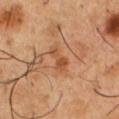Assessment:
Imaged during a routine full-body skin examination; the lesion was not biopsied and no histopathology is available.
Image and clinical context:
Captured under cross-polarized illumination. Automated image analysis of the tile measured a lesion–skin lightness drop of about 9 and a normalized lesion–skin contrast near 6.5. And it measured border irregularity of about 5 on a 0–10 scale, a color-variation rating of about 1.5/10, and radial color variation of about 0.5. Approximately 3.5 mm at its widest. The lesion is located on the chest. A close-up tile cropped from a whole-body skin photograph, about 15 mm across. A male subject aged 48 to 52.